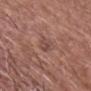The lesion was photographed on a routine skin check and not biopsied; there is no pathology result. The patient is a male in their mid- to late 60s. A roughly 15 mm field-of-view crop from a total-body skin photograph. Captured under white-light illumination. The lesion is located on the head or neck. The recorded lesion diameter is about 3 mm. The total-body-photography lesion software estimated an average lesion color of about L≈46 a*≈20 b*≈23 (CIELAB), about 7 CIELAB-L* units darker than the surrounding skin, and a lesion-to-skin contrast of about 5 (normalized; higher = more distinct). And it measured border irregularity of about 2.5 on a 0–10 scale, a within-lesion color-variation index near 2/10, and radial color variation of about 1. And it measured an automated nevus-likeness rating near 0 out of 100 and lesion-presence confidence of about 85/100.